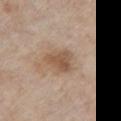Q: Is there a histopathology result?
A: catalogued during a skin exam; not biopsied
Q: Who is the patient?
A: male, approximately 80 years of age
Q: How large is the lesion?
A: ≈3.5 mm
Q: What lighting was used for the tile?
A: white-light illumination
Q: Lesion location?
A: the chest
Q: What is the imaging modality?
A: ~15 mm crop, total-body skin-cancer survey